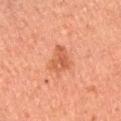Case summary:
• biopsy status · catalogued during a skin exam; not biopsied
• anatomic site · the right upper arm
• patient · female, about 60 years old
• automated lesion analysis · an outline eccentricity of about 0.85 (0 = round, 1 = elongated) and two-axis asymmetry of about 0.4
• diameter · about 4 mm
• image source · total-body-photography crop, ~15 mm field of view
• lighting · cross-polarized